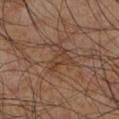{"biopsy_status": "not biopsied; imaged during a skin examination", "automated_metrics": {"area_mm2_approx": 3.5, "shape_asymmetry": 0.45, "cielab_L": 38, "cielab_a": 18, "cielab_b": 28, "vs_skin_darker_L": 6.0, "vs_skin_contrast_norm": 5.5, "color_variation_0_10": 0.5, "peripheral_color_asymmetry": 0.0}, "image": {"source": "total-body photography crop", "field_of_view_mm": 15}, "patient": {"sex": "male", "age_approx": 60}, "site": "left lower leg", "lesion_size": {"long_diameter_mm_approx": 3.0}, "lighting": "cross-polarized"}